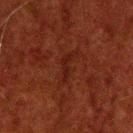Captured during whole-body skin photography for melanoma surveillance; the lesion was not biopsied. On the head or neck. This is a cross-polarized tile. A male patient roughly 60 years of age. An algorithmic analysis of the crop reported an area of roughly 2.5 mm² and a shape eccentricity near 0.95. The software also gave a mean CIELAB color near L≈16 a*≈22 b*≈20 and a lesion-to-skin contrast of about 6 (normalized; higher = more distinct). And it measured a border-irregularity rating of about 6.5/10 and a within-lesion color-variation index near 0/10. The analysis additionally found a classifier nevus-likeness of about 5/100. Measured at roughly 3 mm in maximum diameter. A 15 mm crop from a total-body photograph taken for skin-cancer surveillance.